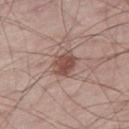Captured during whole-body skin photography for melanoma surveillance; the lesion was not biopsied.
Located on the right thigh.
A male subject, aged 63 to 67.
Imaged with white-light lighting.
The lesion's longest dimension is about 3.5 mm.
The lesion-visualizer software estimated a lesion color around L≈49 a*≈19 b*≈24 in CIELAB, about 12 CIELAB-L* units darker than the surrounding skin, and a normalized lesion–skin contrast near 8.5.
This image is a 15 mm lesion crop taken from a total-body photograph.